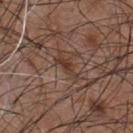Findings:
– follow-up — total-body-photography surveillance lesion; no biopsy
– image source — total-body-photography crop, ~15 mm field of view
– lighting — white-light
– size — about 3.5 mm
– body site — the chest
– image-analysis metrics — a lesion color around L≈37 a*≈18 b*≈25 in CIELAB, about 8 CIELAB-L* units darker than the surrounding skin, and a normalized border contrast of about 7; a border-irregularity rating of about 5/10, a within-lesion color-variation index near 0.5/10, and radial color variation of about 0
– patient — male, aged around 55